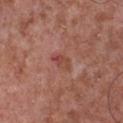Part of a total-body skin-imaging series; this lesion was reviewed on a skin check and was not flagged for biopsy. From the chest. Imaged with white-light lighting. A lesion tile, about 15 mm wide, cut from a 3D total-body photograph. The total-body-photography lesion software estimated a lesion color around L≈46 a*≈26 b*≈26 in CIELAB and a normalized lesion–skin contrast near 6. The software also gave a nevus-likeness score of about 0/100 and a detector confidence of about 100 out of 100 that the crop contains a lesion. The recorded lesion diameter is about 2.5 mm. A male subject approximately 45 years of age.